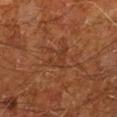• workup — total-body-photography surveillance lesion; no biopsy
• lighting — cross-polarized
• size — ≈3.5 mm
• image-analysis metrics — a footprint of about 4.5 mm², a shape eccentricity near 0.8, and a shape-asymmetry score of about 0.65 (0 = symmetric); a mean CIELAB color near L≈35 a*≈23 b*≈31; a border-irregularity rating of about 8/10 and radial color variation of about 1; a lesion-detection confidence of about 95/100
• subject — male, aged 63 to 67
• location — the left lower leg
• imaging modality — total-body-photography crop, ~15 mm field of view